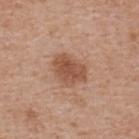Assessment: This lesion was catalogued during total-body skin photography and was not selected for biopsy. Clinical summary: A male subject, aged 58 to 62. The tile uses white-light illumination. The lesion is located on the upper back. The total-body-photography lesion software estimated a border-irregularity index near 2.5/10, internal color variation of about 2.5 on a 0–10 scale, and radial color variation of about 1. Approximately 4 mm at its widest. Cropped from a whole-body photographic skin survey; the tile spans about 15 mm.